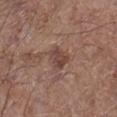The lesion was tiled from a total-body skin photograph and was not biopsied. A male patient aged 58–62. The lesion is on the right lower leg. An algorithmic analysis of the crop reported a lesion color around L≈44 a*≈19 b*≈24 in CIELAB and a normalized border contrast of about 7. And it measured an automated nevus-likeness rating near 80 out of 100 and a detector confidence of about 100 out of 100 that the crop contains a lesion. A region of skin cropped from a whole-body photographic capture, roughly 15 mm wide. The recorded lesion diameter is about 3 mm.